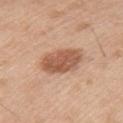Imaged during a routine full-body skin examination; the lesion was not biopsied and no histopathology is available. The patient is a male approximately 70 years of age. A close-up tile cropped from a whole-body skin photograph, about 15 mm across. An algorithmic analysis of the crop reported a lesion area of about 13 mm² and two-axis asymmetry of about 0.15. The software also gave an average lesion color of about L≈56 a*≈22 b*≈32 (CIELAB). And it measured a within-lesion color-variation index near 3.5/10 and a peripheral color-asymmetry measure near 1.5. The analysis additionally found lesion-presence confidence of about 100/100. This is a white-light tile. From the left upper arm. Measured at roughly 4.5 mm in maximum diameter.